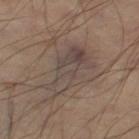No biopsy was performed on this lesion — it was imaged during a full skin examination and was not determined to be concerning.
The tile uses cross-polarized illumination.
The lesion is on the abdomen.
Measured at roughly 7.5 mm in maximum diameter.
Automated image analysis of the tile measured an average lesion color of about L≈42 a*≈11 b*≈19 (CIELAB), a lesion–skin lightness drop of about 7, and a lesion-to-skin contrast of about 6.5 (normalized; higher = more distinct). And it measured border irregularity of about 6.5 on a 0–10 scale. The software also gave a lesion-detection confidence of about 55/100.
The subject is a male aged around 50.
A roughly 15 mm field-of-view crop from a total-body skin photograph.